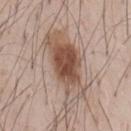Clinical impression: The lesion was photographed on a routine skin check and not biopsied; there is no pathology result. Acquisition and patient details: This image is a 15 mm lesion crop taken from a total-body photograph. The lesion-visualizer software estimated a lesion color around L≈53 a*≈17 b*≈27 in CIELAB and roughly 13 lightness units darker than nearby skin. The software also gave a border-irregularity index near 4/10 and a color-variation rating of about 7/10. It also reported a nevus-likeness score of about 100/100 and a detector confidence of about 100 out of 100 that the crop contains a lesion. A male subject, approximately 55 years of age.